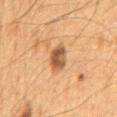Q: Was a biopsy performed?
A: no biopsy performed (imaged during a skin exam)
Q: Illumination type?
A: cross-polarized illumination
Q: What kind of image is this?
A: ~15 mm tile from a whole-body skin photo
Q: Where on the body is the lesion?
A: the mid back
Q: How large is the lesion?
A: about 4 mm
Q: Who is the patient?
A: male, aged around 65
Q: What did automated image analysis measure?
A: a shape eccentricity near 0.85; roughly 12 lightness units darker than nearby skin and a normalized lesion–skin contrast near 9; a border-irregularity index near 2.5/10, a within-lesion color-variation index near 4.5/10, and radial color variation of about 1.5; a nevus-likeness score of about 45/100 and a detector confidence of about 100 out of 100 that the crop contains a lesion